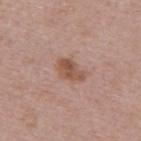Q: Was a biopsy performed?
A: imaged on a skin check; not biopsied
Q: What kind of image is this?
A: total-body-photography crop, ~15 mm field of view
Q: What is the anatomic site?
A: the upper back
Q: Patient demographics?
A: female, aged 58 to 62